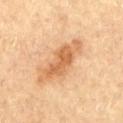Impression:
This lesion was catalogued during total-body skin photography and was not selected for biopsy.
Image and clinical context:
Imaged with cross-polarized lighting. Cropped from a total-body skin-imaging series; the visible field is about 15 mm. The total-body-photography lesion software estimated a lesion color around L≈63 a*≈23 b*≈40 in CIELAB, roughly 11 lightness units darker than nearby skin, and a lesion-to-skin contrast of about 7.5 (normalized; higher = more distinct). Measured at roughly 7.5 mm in maximum diameter. A male patient, aged approximately 85 or older. The lesion is located on the chest.